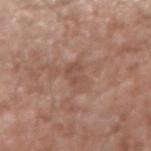workup = no biopsy performed (imaged during a skin exam) | lighting = white-light | body site = the right forearm | lesion size = ~3.5 mm (longest diameter) | acquisition = ~15 mm tile from a whole-body skin photo | subject = male, in their mid- to late 70s.